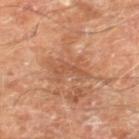This lesion was catalogued during total-body skin photography and was not selected for biopsy. A male subject, in their 70s. A region of skin cropped from a whole-body photographic capture, roughly 15 mm wide. Located on the right lower leg.